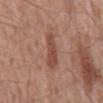Q: Is there a histopathology result?
A: total-body-photography surveillance lesion; no biopsy
Q: What is the imaging modality?
A: 15 mm crop, total-body photography
Q: What did automated image analysis measure?
A: roughly 9 lightness units darker than nearby skin and a normalized lesion–skin contrast near 7; an automated nevus-likeness rating near 50 out of 100 and a detector confidence of about 100 out of 100 that the crop contains a lesion
Q: Illumination type?
A: white-light
Q: Lesion location?
A: the mid back
Q: What are the patient's age and sex?
A: male, aged 58 to 62
Q: What is the lesion's diameter?
A: about 4.5 mm